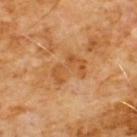Acquisition and patient details:
The lesion's longest dimension is about 4 mm. The subject is a male aged 58–62. Located on the chest. The total-body-photography lesion software estimated a lesion area of about 8 mm², a shape eccentricity near 0.8, and a shape-asymmetry score of about 0.5 (0 = symmetric). The software also gave an automated nevus-likeness rating near 0 out of 100 and lesion-presence confidence of about 100/100. Captured under cross-polarized illumination. A 15 mm crop from a total-body photograph taken for skin-cancer surveillance.